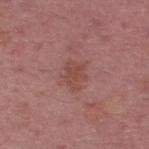Imaged during a routine full-body skin examination; the lesion was not biopsied and no histopathology is available. A male subject in their mid-50s. A close-up tile cropped from a whole-body skin photograph, about 15 mm across. The total-body-photography lesion software estimated a border-irregularity rating of about 3.5/10, a color-variation rating of about 1.5/10, and peripheral color asymmetry of about 0.5. The tile uses white-light illumination. Approximately 3 mm at its widest. The lesion is located on the upper back.